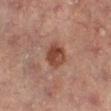Captured during whole-body skin photography for melanoma surveillance; the lesion was not biopsied.
Located on the right lower leg.
Approximately 3 mm at its widest.
A 15 mm close-up extracted from a 3D total-body photography capture.
The patient is a female roughly 70 years of age.
Captured under cross-polarized illumination.